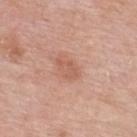Findings:
- workup — total-body-photography surveillance lesion; no biopsy
- imaging modality — total-body-photography crop, ~15 mm field of view
- subject — male, approximately 55 years of age
- size — ≈2.5 mm
- tile lighting — white-light
- anatomic site — the upper back